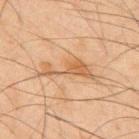This lesion was catalogued during total-body skin photography and was not selected for biopsy. A male subject, in their mid-40s. The lesion's longest dimension is about 6.5 mm. Located on the mid back. The lesion-visualizer software estimated a normalized lesion–skin contrast near 6.5. The software also gave border irregularity of about 8 on a 0–10 scale and internal color variation of about 2.5 on a 0–10 scale. A 15 mm close-up extracted from a 3D total-body photography capture.